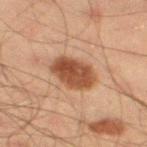{"automated_metrics": {"cielab_L": 41, "cielab_a": 20, "cielab_b": 28, "vs_skin_darker_L": 13.0, "vs_skin_contrast_norm": 10.0, "border_irregularity_0_10": 1.5, "color_variation_0_10": 4.0, "peripheral_color_asymmetry": 1.5}, "lighting": "cross-polarized", "lesion_size": {"long_diameter_mm_approx": 5.0}, "site": "left thigh", "image": {"source": "total-body photography crop", "field_of_view_mm": 15}, "patient": {"sex": "male", "age_approx": 45}}